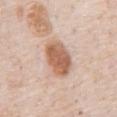Clinical impression:
The lesion was photographed on a routine skin check and not biopsied; there is no pathology result.
Image and clinical context:
The total-body-photography lesion software estimated a lesion area of about 13 mm², a shape eccentricity near 0.8, and two-axis asymmetry of about 0.1. The software also gave a lesion color around L≈61 a*≈21 b*≈31 in CIELAB and a lesion–skin lightness drop of about 14. The software also gave a classifier nevus-likeness of about 90/100 and a lesion-detection confidence of about 100/100. From the abdomen. A region of skin cropped from a whole-body photographic capture, roughly 15 mm wide. A male patient aged approximately 80.